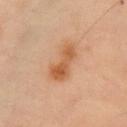  biopsy_status: not biopsied; imaged during a skin examination
  lighting: cross-polarized
  automated_metrics:
    area_mm2_approx: 10.0
    eccentricity: 0.9
    shape_asymmetry: 0.2
    cielab_L: 46
    cielab_a: 19
    cielab_b: 31
    border_irregularity_0_10: 3.0
    color_variation_0_10: 4.0
    peripheral_color_asymmetry: 1.5
  image:
    source: total-body photography crop
    field_of_view_mm: 15
  patient:
    sex: male
    age_approx: 55
  site: chest
  lesion_size:
    long_diameter_mm_approx: 5.5